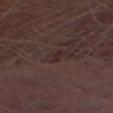Q: Was a biopsy performed?
A: no biopsy performed (imaged during a skin exam)
Q: How was the tile lit?
A: white-light
Q: How was this image acquired?
A: ~15 mm crop, total-body skin-cancer survey
Q: What is the anatomic site?
A: the right thigh
Q: What is the lesion's diameter?
A: ~3 mm (longest diameter)
Q: Patient demographics?
A: male, aged around 70